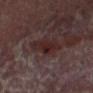Q: Is there a histopathology result?
A: imaged on a skin check; not biopsied
Q: Patient demographics?
A: male, aged approximately 55
Q: How large is the lesion?
A: about 4.5 mm
Q: Automated lesion metrics?
A: a lesion color around L≈21 a*≈16 b*≈14 in CIELAB and roughly 6 lightness units darker than nearby skin; a nevus-likeness score of about 30/100 and a detector confidence of about 95 out of 100 that the crop contains a lesion
Q: What kind of image is this?
A: ~15 mm crop, total-body skin-cancer survey
Q: Lesion location?
A: the left thigh